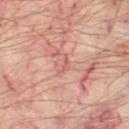This lesion was catalogued during total-body skin photography and was not selected for biopsy.
From the left thigh.
Automated image analysis of the tile measured a footprint of about 2 mm², a shape eccentricity near 0.9, and a shape-asymmetry score of about 0.6 (0 = symmetric). The software also gave a nevus-likeness score of about 0/100.
Imaged with cross-polarized lighting.
A subject in their mid-50s.
A 15 mm close-up tile from a total-body photography series done for melanoma screening.
Measured at roughly 2.5 mm in maximum diameter.